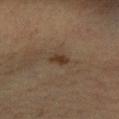Assessment:
No biopsy was performed on this lesion — it was imaged during a full skin examination and was not determined to be concerning.
Clinical summary:
Captured under cross-polarized illumination. A female patient, roughly 45 years of age. An algorithmic analysis of the crop reported a lesion area of about 3 mm² and a shape-asymmetry score of about 0.35 (0 = symmetric). The analysis additionally found a mean CIELAB color near L≈30 a*≈13 b*≈24 and a normalized lesion–skin contrast near 8. The analysis additionally found an automated nevus-likeness rating near 60 out of 100 and lesion-presence confidence of about 100/100. The lesion is on the left forearm. About 2.5 mm across. A roughly 15 mm field-of-view crop from a total-body skin photograph.